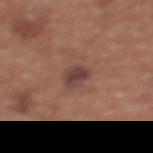Imaged during a routine full-body skin examination; the lesion was not biopsied and no histopathology is available. A roughly 15 mm field-of-view crop from a total-body skin photograph. The lesion is located on the chest. The recorded lesion diameter is about 3 mm. A female subject, aged 33 to 37. This is a white-light tile.